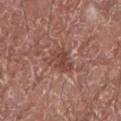Measured at roughly 3.5 mm in maximum diameter. On the right forearm. A close-up tile cropped from a whole-body skin photograph, about 15 mm across. A male patient, aged 68–72.